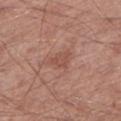Assessment: Part of a total-body skin-imaging series; this lesion was reviewed on a skin check and was not flagged for biopsy. Context: The lesion-visualizer software estimated a lesion–skin lightness drop of about 7 and a normalized border contrast of about 5. It also reported an automated nevus-likeness rating near 0 out of 100 and a detector confidence of about 100 out of 100 that the crop contains a lesion. The tile uses white-light illumination. The lesion is located on the left lower leg. Longest diameter approximately 3 mm. A close-up tile cropped from a whole-body skin photograph, about 15 mm across. The subject is a male roughly 65 years of age.